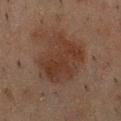biopsy_status: not biopsied; imaged during a skin examination
automated_metrics:
  cielab_L: 27
  cielab_a: 15
  cielab_b: 21
  vs_skin_darker_L: 6.0
  vs_skin_contrast_norm: 7.0
site: front of the torso
patient:
  sex: male
  age_approx: 50
image:
  source: total-body photography crop
  field_of_view_mm: 15
lighting: cross-polarized
lesion_size:
  long_diameter_mm_approx: 6.5